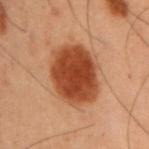Clinical impression: Part of a total-body skin-imaging series; this lesion was reviewed on a skin check and was not flagged for biopsy. Context: A male patient, approximately 55 years of age. This image is a 15 mm lesion crop taken from a total-body photograph. The lesion is located on the right upper arm.